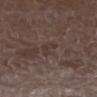| field | value |
|---|---|
| workup | imaged on a skin check; not biopsied |
| illumination | white-light illumination |
| image | ~15 mm tile from a whole-body skin photo |
| size | about 2.5 mm |
| location | the right lower leg |
| patient | male, aged approximately 75 |